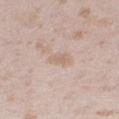The lesion was photographed on a routine skin check and not biopsied; there is no pathology result. A close-up tile cropped from a whole-body skin photograph, about 15 mm across. Approximately 3 mm at its widest. On the right thigh. A female patient in their mid-20s.